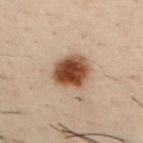follow-up = catalogued during a skin exam; not biopsied
acquisition = ~15 mm tile from a whole-body skin photo
subject = male, aged 28–32
site = the chest
size = ≈4 mm